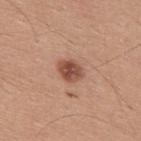Case summary:
• patient — male, approximately 30 years of age
• location — the upper back
• image-analysis metrics — an area of roughly 6 mm² and an outline eccentricity of about 0.6 (0 = round, 1 = elongated); border irregularity of about 1.5 on a 0–10 scale; a classifier nevus-likeness of about 95/100
• lighting — white-light illumination
• size — ≈3 mm
• acquisition — 15 mm crop, total-body photography